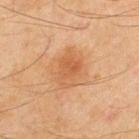<record>
  <biopsy_status>not biopsied; imaged during a skin examination</biopsy_status>
  <patient>
    <sex>male</sex>
    <age_approx>45</age_approx>
  </patient>
  <lighting>cross-polarized</lighting>
  <image>
    <source>total-body photography crop</source>
    <field_of_view_mm>15</field_of_view_mm>
  </image>
  <lesion_size>
    <long_diameter_mm_approx>4.5</long_diameter_mm_approx>
  </lesion_size>
  <site>upper back</site>
</record>